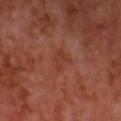Findings:
- notes — total-body-photography surveillance lesion; no biopsy
- location — the head or neck
- image — 15 mm crop, total-body photography
- image-analysis metrics — an average lesion color of about L≈38 a*≈27 b*≈32 (CIELAB) and a lesion-to-skin contrast of about 6 (normalized; higher = more distinct); a border-irregularity index near 6.5/10, a color-variation rating of about 0/10, and peripheral color asymmetry of about 0; a nevus-likeness score of about 0/100
- size — ≈3.5 mm
- tile lighting — cross-polarized illumination
- patient — male, about 55 years old